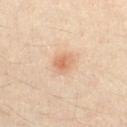Notes:
• workup: no biopsy performed (imaged during a skin exam)
• subject: male, about 35 years old
• tile lighting: cross-polarized illumination
• image: ~15 mm tile from a whole-body skin photo
• size: about 2.5 mm
• body site: the chest